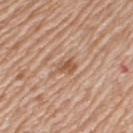Impression: Captured during whole-body skin photography for melanoma surveillance; the lesion was not biopsied. Clinical summary: A female subject, aged around 75. A 15 mm close-up extracted from a 3D total-body photography capture. The lesion is located on the left upper arm.